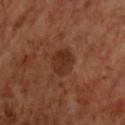This lesion was catalogued during total-body skin photography and was not selected for biopsy.
The lesion-visualizer software estimated an average lesion color of about L≈30 a*≈22 b*≈28 (CIELAB), roughly 7 lightness units darker than nearby skin, and a normalized lesion–skin contrast near 7.5. And it measured a border-irregularity index near 1.5/10, a within-lesion color-variation index near 2.5/10, and radial color variation of about 1. It also reported a nevus-likeness score of about 15/100 and a lesion-detection confidence of about 100/100.
Located on the chest.
A region of skin cropped from a whole-body photographic capture, roughly 15 mm wide.
The tile uses cross-polarized illumination.
A male patient aged 68–72.